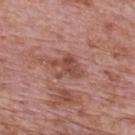Captured during whole-body skin photography for melanoma surveillance; the lesion was not biopsied. Longest diameter approximately 4 mm. A male patient in their 70s. From the mid back. Automated tile analysis of the lesion measured an average lesion color of about L≈48 a*≈23 b*≈26 (CIELAB), roughly 10 lightness units darker than nearby skin, and a normalized lesion–skin contrast near 7. The software also gave a border-irregularity index near 5.5/10, internal color variation of about 2.5 on a 0–10 scale, and a peripheral color-asymmetry measure near 1. A roughly 15 mm field-of-view crop from a total-body skin photograph. Captured under white-light illumination.